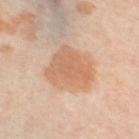| field | value |
|---|---|
| follow-up | catalogued during a skin exam; not biopsied |
| TBP lesion metrics | a footprint of about 18 mm²; a mean CIELAB color near L≈66 a*≈21 b*≈34 and a normalized lesion–skin contrast near 6.5; peripheral color asymmetry of about 1; a nevus-likeness score of about 90/100 and a detector confidence of about 100 out of 100 that the crop contains a lesion |
| patient | female, roughly 50 years of age |
| anatomic site | the right thigh |
| lesion size | ~5 mm (longest diameter) |
| lighting | cross-polarized |
| image | 15 mm crop, total-body photography |